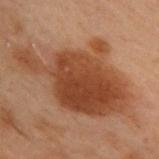The lesion was tiled from a total-body skin photograph and was not biopsied. An algorithmic analysis of the crop reported a shape eccentricity near 0.8. And it measured a classifier nevus-likeness of about 90/100 and lesion-presence confidence of about 100/100. The lesion is on the upper back. The tile uses cross-polarized illumination. A male subject approximately 55 years of age. A 15 mm close-up extracted from a 3D total-body photography capture.